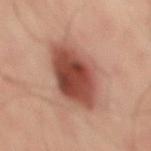notes = total-body-photography surveillance lesion; no biopsy
automated metrics = an area of roughly 27 mm² and an eccentricity of roughly 0.75; a lesion color around L≈48 a*≈25 b*≈28 in CIELAB; lesion-presence confidence of about 100/100
tile lighting = cross-polarized
subject = male, roughly 50 years of age
lesion diameter = about 7 mm
anatomic site = the mid back
imaging modality = ~15 mm crop, total-body skin-cancer survey